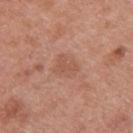Case summary:
- workup · catalogued during a skin exam; not biopsied
- size · ≈3 mm
- imaging modality · ~15 mm tile from a whole-body skin photo
- location · the upper back
- patient · male, aged around 30